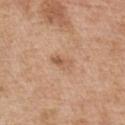Imaged during a routine full-body skin examination; the lesion was not biopsied and no histopathology is available.
This image is a 15 mm lesion crop taken from a total-body photograph.
Approximately 3 mm at its widest.
Imaged with white-light lighting.
The patient is a male aged 53–57.
Automated tile analysis of the lesion measured a border-irregularity index near 2.5/10, internal color variation of about 5 on a 0–10 scale, and a peripheral color-asymmetry measure near 2.
The lesion is on the chest.